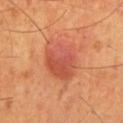biopsy status: no biopsy performed (imaged during a skin exam)
location: the mid back
image: ~15 mm tile from a whole-body skin photo
TBP lesion metrics: a lesion area of about 17 mm² and a shape eccentricity near 0.65; a within-lesion color-variation index near 5.5/10 and radial color variation of about 1.5; a classifier nevus-likeness of about 100/100 and a detector confidence of about 100 out of 100 that the crop contains a lesion
size: about 5 mm
patient: male, about 60 years old
illumination: cross-polarized illumination